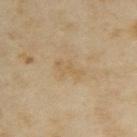The lesion was tiled from a total-body skin photograph and was not biopsied. A female patient, in their mid- to late 30s. Cropped from a total-body skin-imaging series; the visible field is about 15 mm. The lesion is located on the upper back.